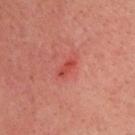Clinical impression: No biopsy was performed on this lesion — it was imaged during a full skin examination and was not determined to be concerning. Context: Cropped from a total-body skin-imaging series; the visible field is about 15 mm. Imaged with cross-polarized lighting. The lesion is located on the head or neck. A male subject aged approximately 55.